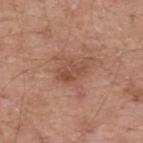{
  "patient": {
    "sex": "male",
    "age_approx": 55
  },
  "site": "upper back",
  "image": {
    "source": "total-body photography crop",
    "field_of_view_mm": 15
  }
}